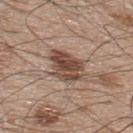<case>
  <biopsy_status>not biopsied; imaged during a skin examination</biopsy_status>
  <patient>
    <sex>male</sex>
    <age_approx>50</age_approx>
  </patient>
  <site>upper back</site>
  <automated_metrics>
    <eccentricity>0.65</eccentricity>
    <border_irregularity_0_10>2.5</border_irregularity_0_10>
    <color_variation_0_10>5.0</color_variation_0_10>
    <peripheral_color_asymmetry>1.5</peripheral_color_asymmetry>
    <nevus_likeness_0_100>85</nevus_likeness_0_100>
  </automated_metrics>
  <lesion_size>
    <long_diameter_mm_approx>4.5</long_diameter_mm_approx>
  </lesion_size>
  <lighting>white-light</lighting>
  <image>
    <source>total-body photography crop</source>
    <field_of_view_mm>15</field_of_view_mm>
  </image>
</case>